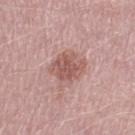Background: A male patient, aged 48 to 52. The lesion's longest dimension is about 4 mm. A close-up tile cropped from a whole-body skin photograph, about 15 mm across. The lesion is on the left thigh.